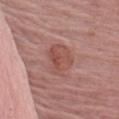<case>
  <biopsy_status>not biopsied; imaged during a skin examination</biopsy_status>
  <site>left upper arm</site>
  <image>
    <source>total-body photography crop</source>
    <field_of_view_mm>15</field_of_view_mm>
  </image>
  <patient>
    <sex>female</sex>
    <age_approx>65</age_approx>
  </patient>
</case>